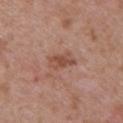workup: imaged on a skin check; not biopsied
lesion diameter: about 3 mm
imaging modality: ~15 mm tile from a whole-body skin photo
tile lighting: white-light
location: the back
subject: female, approximately 30 years of age
image-analysis metrics: an area of roughly 4.5 mm², an eccentricity of roughly 0.85, and a shape-asymmetry score of about 0.25 (0 = symmetric); a lesion–skin lightness drop of about 9 and a normalized border contrast of about 7; internal color variation of about 3 on a 0–10 scale and radial color variation of about 1; a nevus-likeness score of about 0/100 and a detector confidence of about 100 out of 100 that the crop contains a lesion